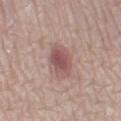{"biopsy_status": "not biopsied; imaged during a skin examination", "patient": {"sex": "female", "age_approx": 60}, "lesion_size": {"long_diameter_mm_approx": 4.0}, "site": "left lower leg", "lighting": "white-light", "image": {"source": "total-body photography crop", "field_of_view_mm": 15}}